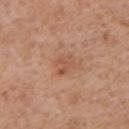Impression:
Part of a total-body skin-imaging series; this lesion was reviewed on a skin check and was not flagged for biopsy.
Context:
The lesion is on the left upper arm. A 15 mm close-up tile from a total-body photography series done for melanoma screening. A male patient approximately 60 years of age. Automated image analysis of the tile measured an area of roughly 3.5 mm², an outline eccentricity of about 0.7 (0 = round, 1 = elongated), and a shape-asymmetry score of about 0.45 (0 = symmetric). The software also gave a border-irregularity index near 5.5/10 and radial color variation of about 0. And it measured a classifier nevus-likeness of about 0/100. Approximately 3 mm at its widest. The tile uses white-light illumination.